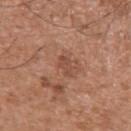notes: catalogued during a skin exam; not biopsied
size: ≈2.5 mm
image-analysis metrics: a footprint of about 3 mm² and two-axis asymmetry of about 0.25; a border-irregularity rating of about 2.5/10 and a peripheral color-asymmetry measure near 0.5; a nevus-likeness score of about 0/100 and a lesion-detection confidence of about 100/100
patient: male, in their mid- to late 70s
site: the right upper arm
image source: 15 mm crop, total-body photography
tile lighting: white-light